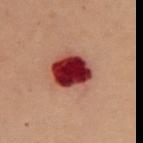Assessment:
The lesion was photographed on a routine skin check and not biopsied; there is no pathology result.
Context:
The recorded lesion diameter is about 4.5 mm. Automated tile analysis of the lesion measured a nevus-likeness score of about 0/100 and a detector confidence of about 100 out of 100 that the crop contains a lesion. Cropped from a total-body skin-imaging series; the visible field is about 15 mm. The tile uses cross-polarized illumination. The subject is a female in their 50s. The lesion is on the chest.